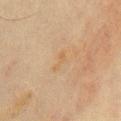  patient:
    sex: male
    age_approx: 35
  lesion_size:
    long_diameter_mm_approx: 2.5
  site: chest
  lighting: cross-polarized
  image:
    source: total-body photography crop
    field_of_view_mm: 15